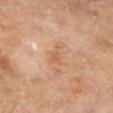This lesion was catalogued during total-body skin photography and was not selected for biopsy. Captured under cross-polarized illumination. The patient is a male aged 63–67. Approximately 3 mm at its widest. A lesion tile, about 15 mm wide, cut from a 3D total-body photograph. The lesion is on the leg.